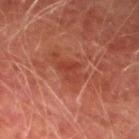The lesion was photographed on a routine skin check and not biopsied; there is no pathology result.
Longest diameter approximately 2.5 mm.
The lesion is located on the left lower leg.
A male patient, in their mid- to late 70s.
Cropped from a total-body skin-imaging series; the visible field is about 15 mm.
Captured under cross-polarized illumination.